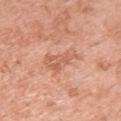Captured during whole-body skin photography for melanoma surveillance; the lesion was not biopsied. About 4.5 mm across. A female subject about 50 years old. Imaged with white-light lighting. A 15 mm close-up tile from a total-body photography series done for melanoma screening. The lesion is on the upper back.